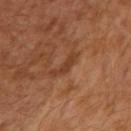The lesion was photographed on a routine skin check and not biopsied; there is no pathology result.